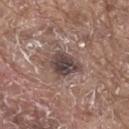<lesion>
<biopsy_status>not biopsied; imaged during a skin examination</biopsy_status>
<site>upper back</site>
<image>
  <source>total-body photography crop</source>
  <field_of_view_mm>15</field_of_view_mm>
</image>
<lighting>white-light</lighting>
<patient>
  <sex>male</sex>
  <age_approx>80</age_approx>
</patient>
</lesion>